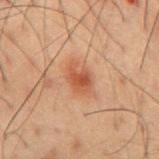Clinical impression:
The lesion was photographed on a routine skin check and not biopsied; there is no pathology result.
Background:
A 15 mm close-up extracted from a 3D total-body photography capture. A male subject, aged 48 to 52. On the mid back.